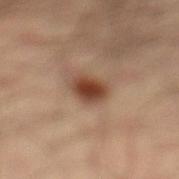- biopsy status: imaged on a skin check; not biopsied
- image source: ~15 mm crop, total-body skin-cancer survey
- location: the leg
- automated lesion analysis: an area of roughly 5.5 mm², an eccentricity of roughly 0.5, and a shape-asymmetry score of about 0.2 (0 = symmetric); a mean CIELAB color near L≈38 a*≈20 b*≈28, about 14 CIELAB-L* units darker than the surrounding skin, and a normalized border contrast of about 11.5; internal color variation of about 3.5 on a 0–10 scale and radial color variation of about 1
- tile lighting: cross-polarized
- subject: male, approximately 35 years of age